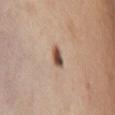<case>
<biopsy_status>not biopsied; imaged during a skin examination</biopsy_status>
<site>front of the torso</site>
<lesion_size>
  <long_diameter_mm_approx>2.5</long_diameter_mm_approx>
</lesion_size>
<patient>
  <sex>female</sex>
  <age_approx>45</age_approx>
</patient>
<image>
  <source>total-body photography crop</source>
  <field_of_view_mm>15</field_of_view_mm>
</image>
<automated_metrics>
  <border_irregularity_0_10>2.5</border_irregularity_0_10>
  <color_variation_0_10>6.0</color_variation_0_10>
  <peripheral_color_asymmetry>2.0</peripheral_color_asymmetry>
  <nevus_likeness_0_100>100</nevus_likeness_0_100>
</automated_metrics>
</case>